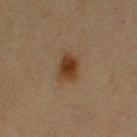<record>
  <biopsy_status>not biopsied; imaged during a skin examination</biopsy_status>
  <site>arm</site>
  <image>
    <source>total-body photography crop</source>
    <field_of_view_mm>15</field_of_view_mm>
  </image>
  <lighting>cross-polarized</lighting>
  <patient>
    <sex>female</sex>
    <age_approx>40</age_approx>
  </patient>
</record>